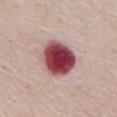Q: Was this lesion biopsied?
A: total-body-photography surveillance lesion; no biopsy
Q: Patient demographics?
A: female, aged around 70
Q: Lesion size?
A: about 5 mm
Q: How was the tile lit?
A: white-light illumination
Q: What is the anatomic site?
A: the abdomen
Q: What is the imaging modality?
A: 15 mm crop, total-body photography